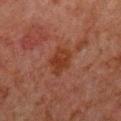The lesion was tiled from a total-body skin photograph and was not biopsied. Located on the chest. A male subject aged 58–62. This is a cross-polarized tile. Approximately 3.5 mm at its widest. A 15 mm close-up extracted from a 3D total-body photography capture.